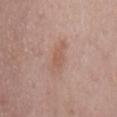  biopsy_status: not biopsied; imaged during a skin examination
  automated_metrics:
    area_mm2_approx: 3.0
    eccentricity: 0.85
    shape_asymmetry: 0.25
    lesion_detection_confidence_0_100: 100
  image:
    source: total-body photography crop
    field_of_view_mm: 15
  patient:
    sex: male
    age_approx: 55
  lighting: white-light
  site: mid back
  lesion_size:
    long_diameter_mm_approx: 2.5